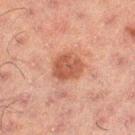This lesion was catalogued during total-body skin photography and was not selected for biopsy.